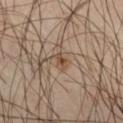No biopsy was performed on this lesion — it was imaged during a full skin examination and was not determined to be concerning. The lesion is on the right thigh. A lesion tile, about 15 mm wide, cut from a 3D total-body photograph. The lesion-visualizer software estimated a footprint of about 3 mm² and a shape-asymmetry score of about 0.4 (0 = symmetric). It also reported a classifier nevus-likeness of about 25/100 and a detector confidence of about 100 out of 100 that the crop contains a lesion. The tile uses cross-polarized illumination. A male subject, aged 63–67.